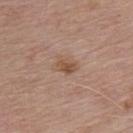Case summary:
• follow-up: catalogued during a skin exam; not biopsied
• illumination: white-light
• patient: male, aged approximately 70
• TBP lesion metrics: a lesion area of about 3.5 mm² and a shape eccentricity near 0.7; an average lesion color of about L≈51 a*≈19 b*≈29 (CIELAB), a lesion–skin lightness drop of about 9, and a normalized border contrast of about 7.5; border irregularity of about 2 on a 0–10 scale, internal color variation of about 2.5 on a 0–10 scale, and peripheral color asymmetry of about 1
• site: the chest
• image source: 15 mm crop, total-body photography
• size: ~2.5 mm (longest diameter)